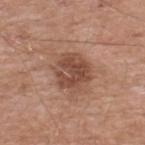<case>
<biopsy_status>not biopsied; imaged during a skin examination</biopsy_status>
<patient>
  <sex>male</sex>
  <age_approx>55</age_approx>
</patient>
<image>
  <source>total-body photography crop</source>
  <field_of_view_mm>15</field_of_view_mm>
</image>
<site>upper back</site>
</case>